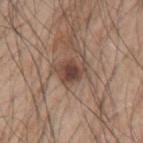Notes:
* site — the left upper arm
* automated lesion analysis — a lesion area of about 7 mm², a shape eccentricity near 0.7, and two-axis asymmetry of about 0.3; a classifier nevus-likeness of about 75/100 and lesion-presence confidence of about 100/100
* patient — male, aged around 50
* diameter — about 3.5 mm
* image — ~15 mm crop, total-body skin-cancer survey